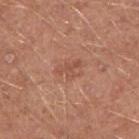Impression: Recorded during total-body skin imaging; not selected for excision or biopsy. Image and clinical context: On the arm. A male patient, in their 40s. Imaged with white-light lighting. Cropped from a total-body skin-imaging series; the visible field is about 15 mm.